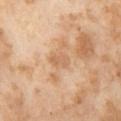Part of a total-body skin-imaging series; this lesion was reviewed on a skin check and was not flagged for biopsy.
A female subject roughly 55 years of age.
A close-up tile cropped from a whole-body skin photograph, about 15 mm across.
The lesion is on the leg.